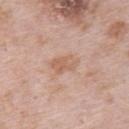Imaged during a routine full-body skin examination; the lesion was not biopsied and no histopathology is available. Captured under white-light illumination. A 15 mm close-up tile from a total-body photography series done for melanoma screening. The recorded lesion diameter is about 3.5 mm. A female subject in their mid-60s. The lesion is on the upper back.